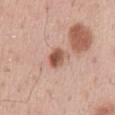This lesion was catalogued during total-body skin photography and was not selected for biopsy. This image is a 15 mm lesion crop taken from a total-body photograph. On the abdomen. A male patient, roughly 55 years of age.